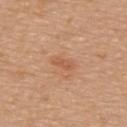<lesion>
<biopsy_status>not biopsied; imaged during a skin examination</biopsy_status>
<patient>
  <sex>male</sex>
  <age_approx>65</age_approx>
</patient>
<lighting>white-light</lighting>
<image>
  <source>total-body photography crop</source>
  <field_of_view_mm>15</field_of_view_mm>
</image>
<site>back</site>
</lesion>